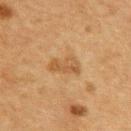Clinical summary: A male patient, aged 73–77. On the upper back. A roughly 15 mm field-of-view crop from a total-body skin photograph.